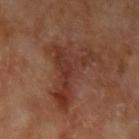Assessment:
No biopsy was performed on this lesion — it was imaged during a full skin examination and was not determined to be concerning.
Context:
This image is a 15 mm lesion crop taken from a total-body photograph. A male patient aged 68–72. An algorithmic analysis of the crop reported a lesion area of about 20 mm² and a symmetry-axis asymmetry near 0.75. And it measured an automated nevus-likeness rating near 0 out of 100 and a lesion-detection confidence of about 100/100. Located on the arm.